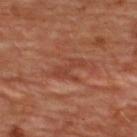Impression:
No biopsy was performed on this lesion — it was imaged during a full skin examination and was not determined to be concerning.